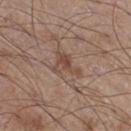<tbp_lesion>
  <biopsy_status>not biopsied; imaged during a skin examination</biopsy_status>
  <patient>
    <sex>male</sex>
    <age_approx>60</age_approx>
  </patient>
  <image>
    <source>total-body photography crop</source>
    <field_of_view_mm>15</field_of_view_mm>
  </image>
  <site>right thigh</site>
</tbp_lesion>